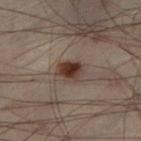Acquisition and patient details:
Automated tile analysis of the lesion measured a mean CIELAB color near L≈28 a*≈14 b*≈19 and a lesion-to-skin contrast of about 11.5 (normalized; higher = more distinct). The analysis additionally found a color-variation rating of about 4.5/10 and a peripheral color-asymmetry measure near 1.5. And it measured a lesion-detection confidence of about 100/100. Imaged with cross-polarized lighting. From the left lower leg. Cropped from a total-body skin-imaging series; the visible field is about 15 mm. A male subject approximately 50 years of age. Approximately 3 mm at its widest.